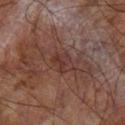Impression:
The lesion was photographed on a routine skin check and not biopsied; there is no pathology result.
Background:
A male patient aged approximately 70. The lesion is located on the right lower leg. A 15 mm crop from a total-body photograph taken for skin-cancer surveillance. The recorded lesion diameter is about 3 mm.